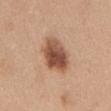Imaged during a routine full-body skin examination; the lesion was not biopsied and no histopathology is available.
The subject is a female aged around 40.
A 15 mm close-up tile from a total-body photography series done for melanoma screening.
The lesion is on the abdomen.